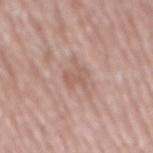Imaged during a routine full-body skin examination; the lesion was not biopsied and no histopathology is available.
The lesion is on the back.
About 3.5 mm across.
A region of skin cropped from a whole-body photographic capture, roughly 15 mm wide.
The patient is a male aged 68–72.